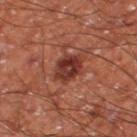The lesion was photographed on a routine skin check and not biopsied; there is no pathology result.
From the right thigh.
A roughly 15 mm field-of-view crop from a total-body skin photograph.
The patient is a male aged around 60.